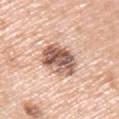Recorded during total-body skin imaging; not selected for excision or biopsy. A male patient, aged 58–62. The total-body-photography lesion software estimated a footprint of about 14 mm², an outline eccentricity of about 0.35 (0 = round, 1 = elongated), and a shape-asymmetry score of about 0.15 (0 = symmetric). The analysis additionally found a mean CIELAB color near L≈59 a*≈21 b*≈27, roughly 18 lightness units darker than nearby skin, and a lesion-to-skin contrast of about 10.5 (normalized; higher = more distinct). About 4.5 mm across. This image is a 15 mm lesion crop taken from a total-body photograph. The lesion is on the left upper arm. This is a white-light tile.